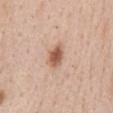Imaged during a routine full-body skin examination; the lesion was not biopsied and no histopathology is available. The lesion-visualizer software estimated an area of roughly 6 mm², an outline eccentricity of about 0.7 (0 = round, 1 = elongated), and two-axis asymmetry of about 0.25. The lesion is located on the abdomen. This image is a 15 mm lesion crop taken from a total-body photograph. The patient is a male aged around 60. Longest diameter approximately 3 mm.